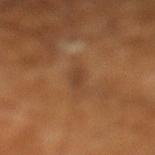workup — total-body-photography surveillance lesion; no biopsy
subject — male, aged 63 to 67
site — the leg
acquisition — ~15 mm tile from a whole-body skin photo
lighting — cross-polarized illumination